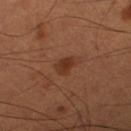Impression: This lesion was catalogued during total-body skin photography and was not selected for biopsy. Clinical summary: The patient is a male aged 48–52. Cropped from a total-body skin-imaging series; the visible field is about 15 mm. On the right thigh.